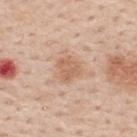Assessment:
Recorded during total-body skin imaging; not selected for excision or biopsy.
Clinical summary:
Measured at roughly 2.5 mm in maximum diameter. A male patient aged around 60. From the upper back. A 15 mm crop from a total-body photograph taken for skin-cancer surveillance. Captured under white-light illumination.